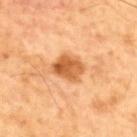Captured during whole-body skin photography for melanoma surveillance; the lesion was not biopsied. From the upper back. A 15 mm crop from a total-body photograph taken for skin-cancer surveillance. A male subject, approximately 60 years of age.